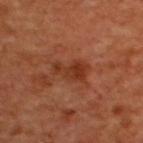Cropped from a total-body skin-imaging series; the visible field is about 15 mm. A male subject aged approximately 50. From the upper back.